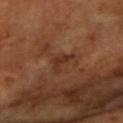  biopsy_status: not biopsied; imaged during a skin examination
  automated_metrics:
    border_irregularity_0_10: 6.0
    color_variation_0_10: 0.5
    nevus_likeness_0_100: 0
    lesion_detection_confidence_0_100: 100
  patient:
    sex: female
    age_approx: 70
  site: right forearm
  image:
    source: total-body photography crop
    field_of_view_mm: 15
  lighting: cross-polarized